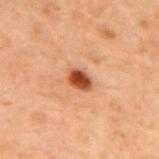Q: Was a biopsy performed?
A: catalogued during a skin exam; not biopsied
Q: Who is the patient?
A: male, in their 70s
Q: What is the lesion's diameter?
A: ~3 mm (longest diameter)
Q: What kind of image is this?
A: 15 mm crop, total-body photography
Q: What is the anatomic site?
A: the upper back
Q: What lighting was used for the tile?
A: cross-polarized illumination